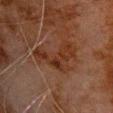Recorded during total-body skin imaging; not selected for excision or biopsy. Imaged with cross-polarized lighting. The total-body-photography lesion software estimated a mean CIELAB color near L≈25 a*≈18 b*≈25 and about 5 CIELAB-L* units darker than the surrounding skin. And it measured internal color variation of about 4 on a 0–10 scale and radial color variation of about 1.5. And it measured lesion-presence confidence of about 100/100. Located on the chest. This image is a 15 mm lesion crop taken from a total-body photograph. Longest diameter approximately 6.5 mm. The subject is a male roughly 80 years of age.